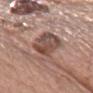Recorded during total-body skin imaging; not selected for excision or biopsy. The subject is a female aged around 60. The lesion-visualizer software estimated a lesion color around L≈49 a*≈18 b*≈24 in CIELAB and a normalized lesion–skin contrast near 8.5. The analysis additionally found a nevus-likeness score of about 5/100. The lesion is on the chest. About 6.5 mm across. A close-up tile cropped from a whole-body skin photograph, about 15 mm across. This is a white-light tile.